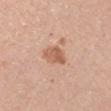Recorded during total-body skin imaging; not selected for excision or biopsy. The subject is a male roughly 40 years of age. The lesion is located on the left upper arm. A roughly 15 mm field-of-view crop from a total-body skin photograph.